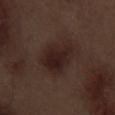<tbp_lesion>
  <biopsy_status>not biopsied; imaged during a skin examination</biopsy_status>
  <site>left thigh</site>
  <lesion_size>
    <long_diameter_mm_approx>5.0</long_diameter_mm_approx>
  </lesion_size>
  <automated_metrics>
    <border_irregularity_0_10>2.5</border_irregularity_0_10>
    <color_variation_0_10>3.5</color_variation_0_10>
    <peripheral_color_asymmetry>1.0</peripheral_color_asymmetry>
    <nevus_likeness_0_100>65</nevus_likeness_0_100>
    <lesion_detection_confidence_0_100>100</lesion_detection_confidence_0_100>
  </automated_metrics>
  <patient>
    <sex>male</sex>
    <age_approx>70</age_approx>
  </patient>
  <image>
    <source>total-body photography crop</source>
    <field_of_view_mm>15</field_of_view_mm>
  </image>
</tbp_lesion>